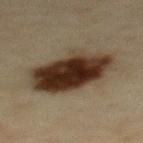notes = no biopsy performed (imaged during a skin exam); image source = ~15 mm tile from a whole-body skin photo; tile lighting = cross-polarized; patient = male, aged 43 to 47; location = the upper back; size = ~8 mm (longest diameter).